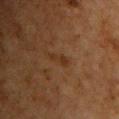Impression:
No biopsy was performed on this lesion — it was imaged during a full skin examination and was not determined to be concerning.
Image and clinical context:
The lesion-visualizer software estimated a footprint of about 2 mm² and an eccentricity of roughly 0.9. It also reported an average lesion color of about L≈26 a*≈17 b*≈27 (CIELAB), a lesion–skin lightness drop of about 5, and a normalized border contrast of about 6. It also reported border irregularity of about 5.5 on a 0–10 scale, internal color variation of about 0 on a 0–10 scale, and radial color variation of about 0. It also reported a nevus-likeness score of about 5/100 and a detector confidence of about 100 out of 100 that the crop contains a lesion. The tile uses cross-polarized illumination. The patient is a male roughly 60 years of age. A 15 mm crop from a total-body photograph taken for skin-cancer surveillance. From the front of the torso. The lesion's longest dimension is about 2.5 mm.